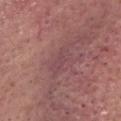| feature | finding |
|---|---|
| follow-up | imaged on a skin check; not biopsied |
| illumination | white-light illumination |
| automated lesion analysis | a footprint of about 4.5 mm² and two-axis asymmetry of about 0.3; an automated nevus-likeness rating near 0 out of 100 |
| anatomic site | the head or neck |
| patient | male, aged 73–77 |
| size | ~3.5 mm (longest diameter) |
| acquisition | ~15 mm tile from a whole-body skin photo |